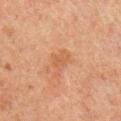Q: Is there a histopathology result?
A: catalogued during a skin exam; not biopsied
Q: Lesion location?
A: the right upper arm
Q: Patient demographics?
A: male, aged 48–52
Q: What is the imaging modality?
A: total-body-photography crop, ~15 mm field of view